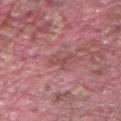follow-up — total-body-photography surveillance lesion; no biopsy | image — ~15 mm tile from a whole-body skin photo | site — the right forearm | subject — male, approximately 40 years of age | image-analysis metrics — a lesion area of about 3 mm², a shape eccentricity near 0.8, and a shape-asymmetry score of about 0.45 (0 = symmetric); a normalized lesion–skin contrast near 5; a border-irregularity index near 5.5/10, a color-variation rating of about 0/10, and radial color variation of about 0.